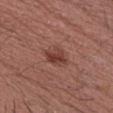Case summary:
– notes · no biopsy performed (imaged during a skin exam)
– image · 15 mm crop, total-body photography
– body site · the chest
– illumination · white-light illumination
– patient · male, approximately 40 years of age
– lesion size · ~2.5 mm (longest diameter)